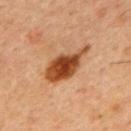Notes:
• location · the upper back
• diameter · ≈6 mm
• patient · male, approximately 45 years of age
• image source · total-body-photography crop, ~15 mm field of view